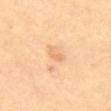The lesion was photographed on a routine skin check and not biopsied; there is no pathology result. Located on the abdomen. The patient is a female approximately 70 years of age. An algorithmic analysis of the crop reported a footprint of about 2 mm², an outline eccentricity of about 0.9 (0 = round, 1 = elongated), and a shape-asymmetry score of about 0.45 (0 = symmetric). It also reported a mean CIELAB color near L≈75 a*≈21 b*≈42, a lesion–skin lightness drop of about 8, and a lesion-to-skin contrast of about 5 (normalized; higher = more distinct). The software also gave a border-irregularity index near 5/10 and internal color variation of about 0 on a 0–10 scale. The recorded lesion diameter is about 2.5 mm. Cropped from a whole-body photographic skin survey; the tile spans about 15 mm. Imaged with cross-polarized lighting.